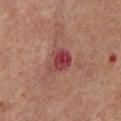Part of a total-body skin-imaging series; this lesion was reviewed on a skin check and was not flagged for biopsy.